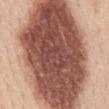biopsy status = catalogued during a skin exam; not biopsied
location = the mid back
lighting = white-light illumination
lesion diameter = about 19 mm
patient = female, in their mid- to late 40s
image-analysis metrics = an area of roughly 130 mm², a shape eccentricity near 0.85, and a symmetry-axis asymmetry near 0.15; a border-irregularity index near 2.5/10, a color-variation rating of about 8/10, and peripheral color asymmetry of about 3; an automated nevus-likeness rating near 95 out of 100
image = ~15 mm tile from a whole-body skin photo